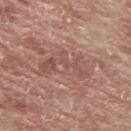This image is a 15 mm lesion crop taken from a total-body photograph. Captured under white-light illumination. From the mid back. Automated tile analysis of the lesion measured a mean CIELAB color near L≈52 a*≈22 b*≈23, about 7 CIELAB-L* units darker than the surrounding skin, and a normalized lesion–skin contrast near 5. The analysis additionally found a nevus-likeness score of about 0/100 and a lesion-detection confidence of about 75/100. Longest diameter approximately 6 mm. The subject is a male roughly 65 years of age.